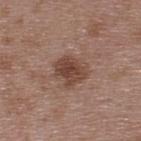The lesion is on the upper back. Captured under white-light illumination. The lesion's longest dimension is about 4 mm. This image is a 15 mm lesion crop taken from a total-body photograph. Automated image analysis of the tile measured a shape eccentricity near 0.6 and two-axis asymmetry of about 0.25. The software also gave an average lesion color of about L≈44 a*≈19 b*≈26 (CIELAB) and about 11 CIELAB-L* units darker than the surrounding skin. It also reported an automated nevus-likeness rating near 35 out of 100. The patient is a male aged 48–52.